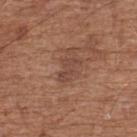The lesion was photographed on a routine skin check and not biopsied; there is no pathology result. Captured under white-light illumination. Cropped from a total-body skin-imaging series; the visible field is about 15 mm. Located on the upper back. About 3 mm across. A male patient aged 73 to 77.